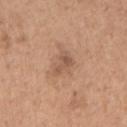The lesion was tiled from a total-body skin photograph and was not biopsied. Cropped from a total-body skin-imaging series; the visible field is about 15 mm. The lesion is located on the right upper arm. A female subject aged around 65.